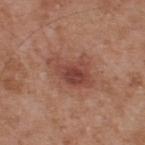Assessment:
Part of a total-body skin-imaging series; this lesion was reviewed on a skin check and was not flagged for biopsy.
Background:
The lesion-visualizer software estimated a lesion area of about 11 mm² and an outline eccentricity of about 0.85 (0 = round, 1 = elongated). The analysis additionally found a lesion color around L≈45 a*≈24 b*≈27 in CIELAB, a lesion–skin lightness drop of about 10, and a lesion-to-skin contrast of about 7.5 (normalized; higher = more distinct). The software also gave a border-irregularity index near 4.5/10, a color-variation rating of about 4.5/10, and peripheral color asymmetry of about 1.5. The lesion's longest dimension is about 5 mm. A male subject, approximately 55 years of age. A 15 mm close-up extracted from a 3D total-body photography capture. The lesion is located on the upper back. Captured under white-light illumination.